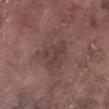The lesion was photographed on a routine skin check and not biopsied; there is no pathology result. A close-up tile cropped from a whole-body skin photograph, about 15 mm across. Captured under white-light illumination. Approximately 4 mm at its widest. Located on the right lower leg. The subject is a male aged 73–77.